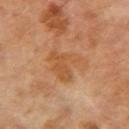This lesion was catalogued during total-body skin photography and was not selected for biopsy. Measured at roughly 4.5 mm in maximum diameter. The patient is a male approximately 70 years of age. Located on the right upper arm. A 15 mm close-up tile from a total-body photography series done for melanoma screening. Captured under cross-polarized illumination.